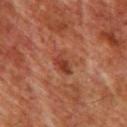The lesion is on the chest. Imaged with cross-polarized lighting. Approximately 3 mm at its widest. A male subject aged 58–62. Cropped from a whole-body photographic skin survey; the tile spans about 15 mm.